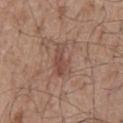The lesion was photographed on a routine skin check and not biopsied; there is no pathology result. A male patient, in their mid-50s. Located on the back. Captured under white-light illumination. Cropped from a whole-body photographic skin survey; the tile spans about 15 mm. Automated tile analysis of the lesion measured an area of roughly 7 mm², an outline eccentricity of about 0.85 (0 = round, 1 = elongated), and a symmetry-axis asymmetry near 0.4. The software also gave an average lesion color of about L≈48 a*≈20 b*≈25 (CIELAB), about 8 CIELAB-L* units darker than the surrounding skin, and a normalized border contrast of about 6.